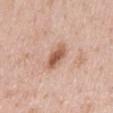This lesion was catalogued during total-body skin photography and was not selected for biopsy. This is a white-light tile. A lesion tile, about 15 mm wide, cut from a 3D total-body photograph. Approximately 3.5 mm at its widest. The lesion is on the mid back. A male subject approximately 50 years of age.